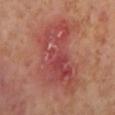Recorded during total-body skin imaging; not selected for excision or biopsy. Measured at roughly 9 mm in maximum diameter. The lesion is located on the left lower leg. A female subject roughly 55 years of age. The lesion-visualizer software estimated an area of roughly 37 mm², a shape eccentricity near 0.8, and a symmetry-axis asymmetry near 0.35. And it measured a border-irregularity index near 4.5/10, a color-variation rating of about 7.5/10, and peripheral color asymmetry of about 2.5. A close-up tile cropped from a whole-body skin photograph, about 15 mm across. Imaged with cross-polarized lighting.